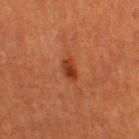follow-up: catalogued during a skin exam; not biopsied
image-analysis metrics: an area of roughly 3.5 mm², an outline eccentricity of about 0.85 (0 = round, 1 = elongated), and a shape-asymmetry score of about 0.3 (0 = symmetric)
imaging modality: ~15 mm crop, total-body skin-cancer survey
illumination: cross-polarized
body site: the right thigh
size: about 2.5 mm
patient: female, aged around 55